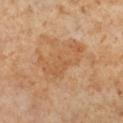{"biopsy_status": "not biopsied; imaged during a skin examination", "image": {"source": "total-body photography crop", "field_of_view_mm": 15}, "lesion_size": {"long_diameter_mm_approx": 6.5}, "automated_metrics": {"area_mm2_approx": 15.0, "shape_asymmetry": 0.35, "border_irregularity_0_10": 5.5, "color_variation_0_10": 3.0, "peripheral_color_asymmetry": 1.0}, "lighting": "cross-polarized", "site": "right lower leg", "patient": {"sex": "female", "age_approx": 50}}